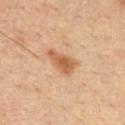Imaged during a routine full-body skin examination; the lesion was not biopsied and no histopathology is available.
A 15 mm crop from a total-body photograph taken for skin-cancer surveillance.
The total-body-photography lesion software estimated a lesion–skin lightness drop of about 11 and a normalized lesion–skin contrast near 8. It also reported radial color variation of about 0.5.
The lesion is located on the front of the torso.
Approximately 4 mm at its widest.
A male subject, aged approximately 35.